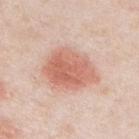Q: Was this lesion biopsied?
A: catalogued during a skin exam; not biopsied
Q: What is the imaging modality?
A: 15 mm crop, total-body photography
Q: What lighting was used for the tile?
A: white-light illumination
Q: Automated lesion metrics?
A: a lesion area of about 21 mm², an eccentricity of roughly 0.7, and two-axis asymmetry of about 0.15; a lesion-detection confidence of about 100/100
Q: Who is the patient?
A: male, aged 48 to 52
Q: What is the anatomic site?
A: the chest